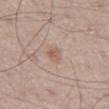• workup: catalogued during a skin exam; not biopsied
• site: the chest
• patient: male, aged approximately 65
• tile lighting: white-light illumination
• diameter: about 2.5 mm
• imaging modality: 15 mm crop, total-body photography
• image-analysis metrics: an average lesion color of about L≈59 a*≈17 b*≈27 (CIELAB) and a lesion–skin lightness drop of about 7; a within-lesion color-variation index near 2.5/10